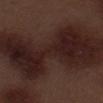Part of a total-body skin-imaging series; this lesion was reviewed on a skin check and was not flagged for biopsy. The tile uses white-light illumination. The patient is a male in their 70s. A roughly 15 mm field-of-view crop from a total-body skin photograph. Located on the left thigh. Automated image analysis of the tile measured a peripheral color-asymmetry measure near 1.5.